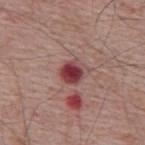Assessment: Part of a total-body skin-imaging series; this lesion was reviewed on a skin check and was not flagged for biopsy. Context: An algorithmic analysis of the crop reported a shape eccentricity near 0.4 and a symmetry-axis asymmetry near 0.15. The software also gave a lesion color around L≈42 a*≈28 b*≈20 in CIELAB, about 15 CIELAB-L* units darker than the surrounding skin, and a normalized lesion–skin contrast near 11. The analysis additionally found a border-irregularity index near 1.5/10 and peripheral color asymmetry of about 2. The lesion is located on the upper back. A male patient aged 63–67. Longest diameter approximately 3 mm. A lesion tile, about 15 mm wide, cut from a 3D total-body photograph. Captured under white-light illumination.